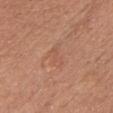workup: imaged on a skin check; not biopsied
patient: female, aged approximately 55
image source: 15 mm crop, total-body photography
size: about 3 mm
tile lighting: white-light
automated lesion analysis: a footprint of about 4.5 mm²; an average lesion color of about L≈55 a*≈23 b*≈31 (CIELAB), roughly 5 lightness units darker than nearby skin, and a lesion-to-skin contrast of about 3.5 (normalized; higher = more distinct)
body site: the head or neck